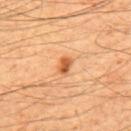Image and clinical context: The lesion's longest dimension is about 2.5 mm. A roughly 15 mm field-of-view crop from a total-body skin photograph. The total-body-photography lesion software estimated a lesion color around L≈57 a*≈27 b*≈41 in CIELAB, roughly 13 lightness units darker than nearby skin, and a normalized border contrast of about 8.5. It also reported border irregularity of about 2.5 on a 0–10 scale and a within-lesion color-variation index near 3.5/10. On the upper back. Captured under cross-polarized illumination. A male patient, aged 48–52.